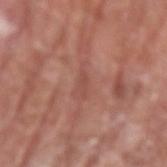Part of a total-body skin-imaging series; this lesion was reviewed on a skin check and was not flagged for biopsy.
Located on the left upper arm.
A region of skin cropped from a whole-body photographic capture, roughly 15 mm wide.
A male patient approximately 65 years of age.
The recorded lesion diameter is about 2.5 mm.
The lesion-visualizer software estimated a lesion area of about 2 mm², a shape eccentricity near 0.95, and a symmetry-axis asymmetry near 0.4. The software also gave a lesion-to-skin contrast of about 5 (normalized; higher = more distinct). The analysis additionally found internal color variation of about 0 on a 0–10 scale. It also reported an automated nevus-likeness rating near 0 out of 100 and a lesion-detection confidence of about 55/100.